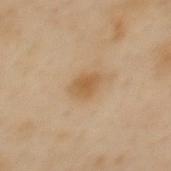Imaged during a routine full-body skin examination; the lesion was not biopsied and no histopathology is available. A female patient, roughly 60 years of age. The lesion is located on the back. This is a cross-polarized tile. Cropped from a total-body skin-imaging series; the visible field is about 15 mm.